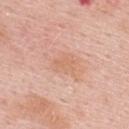Recorded during total-body skin imaging; not selected for excision or biopsy. A roughly 15 mm field-of-view crop from a total-body skin photograph. Located on the upper back. A male subject in their mid- to late 50s. Automated tile analysis of the lesion measured a border-irregularity index near 4/10, a within-lesion color-variation index near 1.5/10, and radial color variation of about 0.5. The software also gave a nevus-likeness score of about 0/100 and a detector confidence of about 100 out of 100 that the crop contains a lesion. The lesion's longest dimension is about 3 mm.